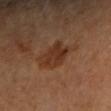Findings:
- notes — no biopsy performed (imaged during a skin exam)
- body site — the left forearm
- patient — female, aged around 65
- imaging modality — 15 mm crop, total-body photography